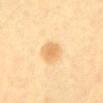follow-up: total-body-photography surveillance lesion; no biopsy
image-analysis metrics: a border-irregularity index near 1.5/10, internal color variation of about 2 on a 0–10 scale, and peripheral color asymmetry of about 0.5; a classifier nevus-likeness of about 75/100 and a detector confidence of about 100 out of 100 that the crop contains a lesion
lesion size: ~3 mm (longest diameter)
anatomic site: the front of the torso
subject: aged around 60
tile lighting: cross-polarized illumination
imaging modality: ~15 mm tile from a whole-body skin photo